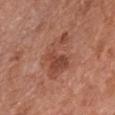| feature | finding |
|---|---|
| workup | catalogued during a skin exam; not biopsied |
| location | the front of the torso |
| image | ~15 mm crop, total-body skin-cancer survey |
| patient | female, aged around 65 |